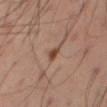No biopsy was performed on this lesion — it was imaged during a full skin examination and was not determined to be concerning. A 15 mm close-up tile from a total-body photography series done for melanoma screening. The subject is a male aged approximately 60. About 2 mm across. The lesion is on the leg.